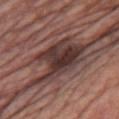Q: Is there a histopathology result?
A: imaged on a skin check; not biopsied
Q: Who is the patient?
A: male, in their mid-80s or older
Q: What is the imaging modality?
A: 15 mm crop, total-body photography
Q: Lesion location?
A: the chest
Q: Lesion size?
A: about 9.5 mm
Q: What lighting was used for the tile?
A: white-light illumination